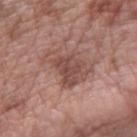This lesion was catalogued during total-body skin photography and was not selected for biopsy. The recorded lesion diameter is about 5.5 mm. An algorithmic analysis of the crop reported an area of roughly 13 mm² and a symmetry-axis asymmetry near 0.45. The software also gave border irregularity of about 5.5 on a 0–10 scale, a color-variation rating of about 3.5/10, and radial color variation of about 1. The software also gave a nevus-likeness score of about 5/100 and lesion-presence confidence of about 100/100. Captured under white-light illumination. A 15 mm close-up extracted from a 3D total-body photography capture. A female patient roughly 60 years of age. Located on the left forearm.